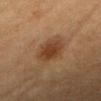The lesion was tiled from a total-body skin photograph and was not biopsied.
On the head or neck.
A female patient aged around 45.
Cropped from a whole-body photographic skin survey; the tile spans about 15 mm.